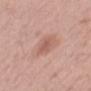Assessment:
No biopsy was performed on this lesion — it was imaged during a full skin examination and was not determined to be concerning.
Acquisition and patient details:
A male patient aged around 55. Automated image analysis of the tile measured an area of roughly 5 mm² and a shape-asymmetry score of about 0.2 (0 = symmetric). The software also gave roughly 9 lightness units darker than nearby skin and a normalized border contrast of about 6. The lesion's longest dimension is about 3.5 mm. Imaged with white-light lighting. A lesion tile, about 15 mm wide, cut from a 3D total-body photograph. On the mid back.